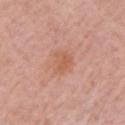image:
  source: total-body photography crop
  field_of_view_mm: 15
lighting: white-light
lesion_size:
  long_diameter_mm_approx: 3.0
site: left upper arm
patient:
  sex: female
  age_approx: 65